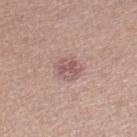Clinical summary: Captured under white-light illumination. The total-body-photography lesion software estimated an area of roughly 6.5 mm² and two-axis asymmetry of about 0.15. And it measured a classifier nevus-likeness of about 0/100 and lesion-presence confidence of about 100/100. Located on the right lower leg. Measured at roughly 3 mm in maximum diameter. A female patient aged 18–22. This image is a 15 mm lesion crop taken from a total-body photograph.